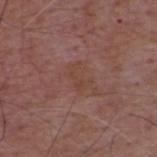Impression: Captured during whole-body skin photography for melanoma surveillance; the lesion was not biopsied. Clinical summary: The total-body-photography lesion software estimated a shape eccentricity near 0.85 and two-axis asymmetry of about 0.55. The tile uses white-light illumination. The subject is a male aged 48 to 52. A roughly 15 mm field-of-view crop from a total-body skin photograph. The lesion is located on the upper back.